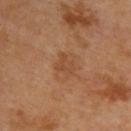{"biopsy_status": "not biopsied; imaged during a skin examination", "automated_metrics": {"area_mm2_approx": 4.0, "shape_asymmetry": 0.5, "vs_skin_darker_L": 6.0, "vs_skin_contrast_norm": 5.0, "border_irregularity_0_10": 4.5, "color_variation_0_10": 1.5, "peripheral_color_asymmetry": 0.5, "nevus_likeness_0_100": 0, "lesion_detection_confidence_0_100": 100}, "lighting": "cross-polarized", "image": {"source": "total-body photography crop", "field_of_view_mm": 15}, "site": "back", "lesion_size": {"long_diameter_mm_approx": 3.0}, "patient": {"sex": "male", "age_approx": 70}}